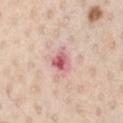Q: Was a biopsy performed?
A: imaged on a skin check; not biopsied
Q: Lesion size?
A: about 3 mm
Q: What is the imaging modality?
A: ~15 mm crop, total-body skin-cancer survey
Q: Who is the patient?
A: male, aged 58 to 62
Q: What is the anatomic site?
A: the chest